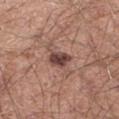Clinical impression:
Imaged during a routine full-body skin examination; the lesion was not biopsied and no histopathology is available.
Context:
Cropped from a whole-body photographic skin survey; the tile spans about 15 mm. Longest diameter approximately 2.5 mm. The lesion is located on the left thigh. A male patient, roughly 40 years of age. The lesion-visualizer software estimated a symmetry-axis asymmetry near 0.2. The analysis additionally found an average lesion color of about L≈42 a*≈20 b*≈22 (CIELAB), about 14 CIELAB-L* units darker than the surrounding skin, and a normalized border contrast of about 11. And it measured border irregularity of about 1.5 on a 0–10 scale and peripheral color asymmetry of about 1. And it measured a nevus-likeness score of about 85/100 and a lesion-detection confidence of about 100/100.